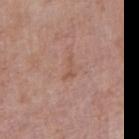Assessment:
Captured during whole-body skin photography for melanoma surveillance; the lesion was not biopsied.
Acquisition and patient details:
Approximately 3 mm at its widest. A close-up tile cropped from a whole-body skin photograph, about 15 mm across. Located on the right upper arm. The patient is a male aged around 55. An algorithmic analysis of the crop reported an outline eccentricity of about 0.9 (0 = round, 1 = elongated) and two-axis asymmetry of about 0.6. The software also gave a lesion color around L≈54 a*≈21 b*≈28 in CIELAB and a normalized lesion–skin contrast near 5. The software also gave a border-irregularity index near 7/10, a within-lesion color-variation index near 0/10, and peripheral color asymmetry of about 0. Imaged with white-light lighting.